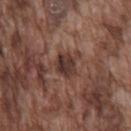The lesion was tiled from a total-body skin photograph and was not biopsied. The lesion-visualizer software estimated a lesion area of about 6 mm², an eccentricity of roughly 0.55, and two-axis asymmetry of about 0.25. And it measured an average lesion color of about L≈35 a*≈18 b*≈22 (CIELAB) and a lesion–skin lightness drop of about 10. The analysis additionally found a nevus-likeness score of about 0/100 and a detector confidence of about 95 out of 100 that the crop contains a lesion. About 3 mm across. A male subject, about 75 years old. Imaged with white-light lighting. The lesion is on the front of the torso. Cropped from a whole-body photographic skin survey; the tile spans about 15 mm.